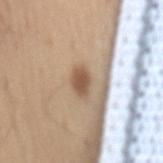Clinical impression: Imaged during a routine full-body skin examination; the lesion was not biopsied and no histopathology is available. Acquisition and patient details: An algorithmic analysis of the crop reported an area of roughly 5 mm², an eccentricity of roughly 0.8, and a shape-asymmetry score of about 0.25 (0 = symmetric). The analysis additionally found a lesion color around L≈55 a*≈17 b*≈30 in CIELAB. It also reported a border-irregularity rating of about 2.5/10, a color-variation rating of about 3/10, and peripheral color asymmetry of about 1. The recorded lesion diameter is about 3.5 mm. The subject is a male aged around 55. Located on the mid back. The tile uses white-light illumination. This image is a 15 mm lesion crop taken from a total-body photograph.